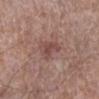This lesion was catalogued during total-body skin photography and was not selected for biopsy. The lesion is on the leg. The subject is a male aged approximately 55. The lesion's longest dimension is about 4 mm. Captured under white-light illumination. A 15 mm close-up extracted from a 3D total-body photography capture.